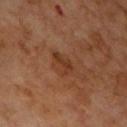Part of a total-body skin-imaging series; this lesion was reviewed on a skin check and was not flagged for biopsy. The tile uses cross-polarized illumination. The subject is a male approximately 65 years of age. This image is a 15 mm lesion crop taken from a total-body photograph. Located on the front of the torso.